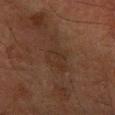| feature | finding |
|---|---|
| biopsy status | imaged on a skin check; not biopsied |
| imaging modality | ~15 mm tile from a whole-body skin photo |
| diameter | ≈4 mm |
| image-analysis metrics | a mean CIELAB color near L≈23 a*≈13 b*≈21, a lesion–skin lightness drop of about 3, and a lesion-to-skin contrast of about 4.5 (normalized; higher = more distinct); internal color variation of about 2 on a 0–10 scale and radial color variation of about 0.5; lesion-presence confidence of about 100/100 |
| patient | male, aged around 60 |
| anatomic site | the right forearm |